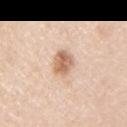biopsy status: catalogued during a skin exam; not biopsied
subject: male, in their mid- to late 40s
automated lesion analysis: a lesion area of about 6 mm²; an average lesion color of about L≈65 a*≈20 b*≈32 (CIELAB), roughly 14 lightness units darker than nearby skin, and a normalized border contrast of about 8.5; a within-lesion color-variation index near 4.5/10 and a peripheral color-asymmetry measure near 1.5
size: ≈3 mm
illumination: white-light
acquisition: ~15 mm tile from a whole-body skin photo
anatomic site: the right upper arm